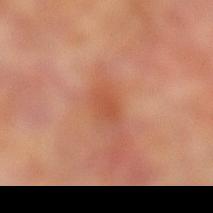Findings:
– biopsy status — total-body-photography surveillance lesion; no biopsy
– imaging modality — total-body-photography crop, ~15 mm field of view
– patient — male, aged 68 to 72
– anatomic site — the left lower leg
– automated metrics — roughly 7 lightness units darker than nearby skin and a lesion-to-skin contrast of about 5.5 (normalized; higher = more distinct); a nevus-likeness score of about 0/100 and lesion-presence confidence of about 100/100
– tile lighting — cross-polarized
– lesion size — ~3 mm (longest diameter)